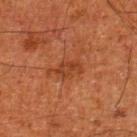Captured during whole-body skin photography for melanoma surveillance; the lesion was not biopsied.
The patient is a male about 80 years old.
This image is a 15 mm lesion crop taken from a total-body photograph.
Located on the leg.
The lesion's longest dimension is about 3 mm.